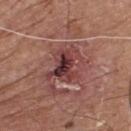Case summary:
• follow-up — no biopsy performed (imaged during a skin exam)
• patient — male, in their 80s
• site — the chest
• size — ~5 mm (longest diameter)
• acquisition — ~15 mm crop, total-body skin-cancer survey